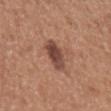Impression: Captured during whole-body skin photography for melanoma surveillance; the lesion was not biopsied. Image and clinical context: The tile uses white-light illumination. The patient is a female aged around 50. About 3 mm across. From the left upper arm. Cropped from a whole-body photographic skin survey; the tile spans about 15 mm.